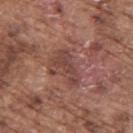No biopsy was performed on this lesion — it was imaged during a full skin examination and was not determined to be concerning. A male patient, aged 73–77. On the upper back. The recorded lesion diameter is about 4 mm. A 15 mm close-up tile from a total-body photography series done for melanoma screening.